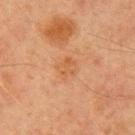  biopsy_status: not biopsied; imaged during a skin examination